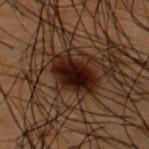notes = total-body-photography surveillance lesion; no biopsy | anatomic site = the upper back | image = ~15 mm tile from a whole-body skin photo | patient = male, aged around 50.